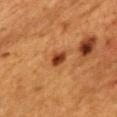Q: Is there a histopathology result?
A: no biopsy performed (imaged during a skin exam)
Q: Lesion size?
A: ~2 mm (longest diameter)
Q: Lesion location?
A: the mid back
Q: How was the tile lit?
A: cross-polarized
Q: Patient demographics?
A: female, aged around 55
Q: How was this image acquired?
A: ~15 mm tile from a whole-body skin photo
Q: What did automated image analysis measure?
A: a mean CIELAB color near L≈34 a*≈24 b*≈33, a lesion–skin lightness drop of about 13, and a normalized lesion–skin contrast near 11; a border-irregularity index near 2.5/10 and a peripheral color-asymmetry measure near 1